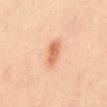The tile uses cross-polarized illumination. Cropped from a whole-body photographic skin survey; the tile spans about 15 mm. On the back. Automated tile analysis of the lesion measured a lesion area of about 5 mm² and a symmetry-axis asymmetry near 0.2. The software also gave a within-lesion color-variation index near 3/10. A female patient, aged approximately 40.